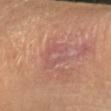notes: imaged on a skin check; not biopsied | subject: male, aged approximately 60 | body site: the arm | image source: total-body-photography crop, ~15 mm field of view.